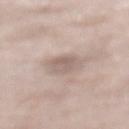Impression: This lesion was catalogued during total-body skin photography and was not selected for biopsy. Context: About 3.5 mm across. Cropped from a whole-body photographic skin survey; the tile spans about 15 mm. Located on the upper back. The patient is a male aged around 85 or older. The tile uses white-light illumination.